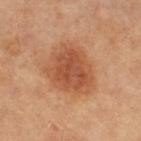<tbp_lesion>
<biopsy_status>not biopsied; imaged during a skin examination</biopsy_status>
<site>right thigh</site>
<patient>
  <sex>female</sex>
  <age_approx>60</age_approx>
</patient>
<image>
  <source>total-body photography crop</source>
  <field_of_view_mm>15</field_of_view_mm>
</image>
<automated_metrics>
  <cielab_L>53</cielab_L>
  <cielab_a>26</cielab_a>
  <cielab_b>37</cielab_b>
  <vs_skin_darker_L>11.0</vs_skin_darker_L>
  <vs_skin_contrast_norm>7.5</vs_skin_contrast_norm>
  <border_irregularity_0_10>2.5</border_irregularity_0_10>
  <peripheral_color_asymmetry>1.5</peripheral_color_asymmetry>
  <nevus_likeness_0_100>100</nevus_likeness_0_100>
  <lesion_detection_confidence_0_100>100</lesion_detection_confidence_0_100>
</automated_metrics>
</tbp_lesion>